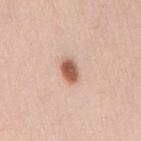Captured during whole-body skin photography for melanoma surveillance; the lesion was not biopsied.
The patient is a male approximately 35 years of age.
A lesion tile, about 15 mm wide, cut from a 3D total-body photograph.
This is a white-light tile.
The lesion is located on the mid back.
The total-body-photography lesion software estimated an average lesion color of about L≈57 a*≈23 b*≈31 (CIELAB) and about 17 CIELAB-L* units darker than the surrounding skin. The analysis additionally found a border-irregularity rating of about 1.5/10, internal color variation of about 3.5 on a 0–10 scale, and a peripheral color-asymmetry measure near 1. And it measured an automated nevus-likeness rating near 100 out of 100 and lesion-presence confidence of about 100/100.